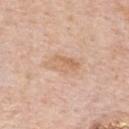Case summary:
* anatomic site: the upper back
* TBP lesion metrics: a lesion area of about 8.5 mm² and a shape-asymmetry score of about 0.25 (0 = symmetric); a within-lesion color-variation index near 4.5/10 and a peripheral color-asymmetry measure near 1.5
* image: ~15 mm crop, total-body skin-cancer survey
* subject: male, aged approximately 60